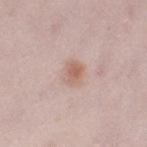Impression: No biopsy was performed on this lesion — it was imaged during a full skin examination and was not determined to be concerning. Acquisition and patient details: A female patient aged approximately 30. Automated image analysis of the tile measured a footprint of about 5 mm², a shape eccentricity near 0.65, and a shape-asymmetry score of about 0.2 (0 = symmetric). The analysis additionally found a lesion color around L≈62 a*≈19 b*≈26 in CIELAB, roughly 9 lightness units darker than nearby skin, and a normalized border contrast of about 6.5. The software also gave a border-irregularity index near 2/10, a color-variation rating of about 4/10, and radial color variation of about 1. Located on the left thigh. A 15 mm close-up extracted from a 3D total-body photography capture.